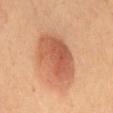{"biopsy_status": "not biopsied; imaged during a skin examination", "lesion_size": {"long_diameter_mm_approx": 6.5}, "lighting": "cross-polarized", "patient": {"sex": "female"}, "automated_metrics": {"eccentricity": 0.7, "shape_asymmetry": 0.15}, "image": {"source": "total-body photography crop", "field_of_view_mm": 15}, "site": "lower back"}